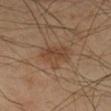Impression:
This lesion was catalogued during total-body skin photography and was not selected for biopsy.
Clinical summary:
From the right lower leg. A close-up tile cropped from a whole-body skin photograph, about 15 mm across. This is a cross-polarized tile. A male subject aged around 70.